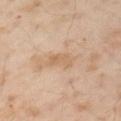biopsy status — no biopsy performed (imaged during a skin exam) | imaging modality — ~15 mm tile from a whole-body skin photo | lesion size — ~3 mm (longest diameter) | subject — male, aged around 55 | location — the left upper arm | illumination — cross-polarized illumination | automated lesion analysis — a footprint of about 4.5 mm², a shape eccentricity near 0.8, and two-axis asymmetry of about 0.3; a lesion color around L≈63 a*≈17 b*≈34 in CIELAB, roughly 6 lightness units darker than nearby skin, and a lesion-to-skin contrast of about 5 (normalized; higher = more distinct).